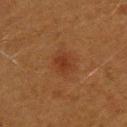Captured during whole-body skin photography for melanoma surveillance; the lesion was not biopsied.
A female patient in their 40s.
This image is a 15 mm lesion crop taken from a total-body photograph.
The lesion is on the upper back.